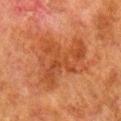Impression: Recorded during total-body skin imaging; not selected for excision or biopsy. Context: The patient is a male roughly 80 years of age. Captured under cross-polarized illumination. Automated image analysis of the tile measured an eccentricity of roughly 0.8. The software also gave a mean CIELAB color near L≈39 a*≈24 b*≈33, about 7 CIELAB-L* units darker than the surrounding skin, and a normalized border contrast of about 6. And it measured a border-irregularity rating of about 7/10, a color-variation rating of about 4/10, and radial color variation of about 1.5. It also reported an automated nevus-likeness rating near 0 out of 100 and lesion-presence confidence of about 100/100. Longest diameter approximately 8 mm. Cropped from a total-body skin-imaging series; the visible field is about 15 mm. The lesion is located on the right lower leg.